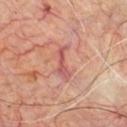Impression: Imaged during a routine full-body skin examination; the lesion was not biopsied and no histopathology is available. Acquisition and patient details: Captured under cross-polarized illumination. Located on the chest. This image is a 15 mm lesion crop taken from a total-body photograph. A male subject, aged approximately 60. Approximately 4 mm at its widest.